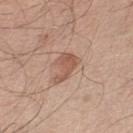Captured during whole-body skin photography for melanoma surveillance; the lesion was not biopsied.
A 15 mm crop from a total-body photograph taken for skin-cancer surveillance.
Measured at roughly 4 mm in maximum diameter.
The subject is a male about 50 years old.
The lesion is on the left lower leg.
This is a white-light tile.